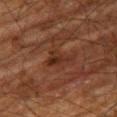Q: Is there a histopathology result?
A: imaged on a skin check; not biopsied
Q: How large is the lesion?
A: ≈3.5 mm
Q: Where on the body is the lesion?
A: the right thigh
Q: What is the imaging modality?
A: ~15 mm tile from a whole-body skin photo
Q: Who is the patient?
A: male, aged approximately 80
Q: Automated lesion metrics?
A: a mean CIELAB color near L≈23 a*≈18 b*≈23, a lesion–skin lightness drop of about 6, and a normalized lesion–skin contrast near 6.5; a border-irregularity index near 5.5/10 and a color-variation rating of about 2.5/10